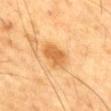notes: imaged on a skin check; not biopsied | imaging modality: 15 mm crop, total-body photography | patient: male, aged approximately 85 or older | size: ≈3.5 mm | body site: the chest | automated metrics: a footprint of about 7 mm², a shape eccentricity near 0.7, and a symmetry-axis asymmetry near 0.2.